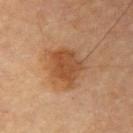Impression:
Imaged during a routine full-body skin examination; the lesion was not biopsied and no histopathology is available.
Clinical summary:
The lesion is located on the right upper arm. The tile uses cross-polarized illumination. A male subject aged 83 or older. Automated image analysis of the tile measured border irregularity of about 2.5 on a 0–10 scale, a color-variation rating of about 3.5/10, and peripheral color asymmetry of about 1. The analysis additionally found an automated nevus-likeness rating near 95 out of 100 and a lesion-detection confidence of about 100/100. A 15 mm close-up extracted from a 3D total-body photography capture.